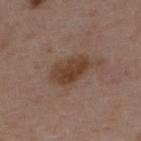Clinical impression:
Part of a total-body skin-imaging series; this lesion was reviewed on a skin check and was not flagged for biopsy.
Clinical summary:
A female subject, aged around 45. Located on the upper back. Measured at roughly 5 mm in maximum diameter. A close-up tile cropped from a whole-body skin photograph, about 15 mm across.